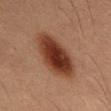follow-up=catalogued during a skin exam; not biopsied | acquisition=total-body-photography crop, ~15 mm field of view | tile lighting=cross-polarized illumination | anatomic site=the mid back | patient=male, aged 53 to 57 | lesion diameter=about 6.5 mm | TBP lesion metrics=a color-variation rating of about 5.5/10 and peripheral color asymmetry of about 1.5; an automated nevus-likeness rating near 100 out of 100 and a detector confidence of about 100 out of 100 that the crop contains a lesion.